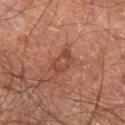Clinical impression: Imaged during a routine full-body skin examination; the lesion was not biopsied and no histopathology is available. Acquisition and patient details: Cropped from a total-body skin-imaging series; the visible field is about 15 mm. The total-body-photography lesion software estimated a lesion area of about 6 mm², an eccentricity of roughly 0.75, and two-axis asymmetry of about 0.25. The analysis additionally found a border-irregularity rating of about 2.5/10. A male subject, aged around 60. Longest diameter approximately 3.5 mm. The lesion is located on the right leg.